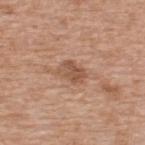biopsy_status: not biopsied; imaged during a skin examination
image:
  source: total-body photography crop
  field_of_view_mm: 15
automated_metrics:
  eccentricity: 0.75
  shape_asymmetry: 0.25
  cielab_L: 53
  cielab_a: 21
  cielab_b: 31
  vs_skin_darker_L: 10.0
  vs_skin_contrast_norm: 7.0
lighting: white-light
lesion_size:
  long_diameter_mm_approx: 3.0
site: upper back
patient:
  sex: male
  age_approx: 55